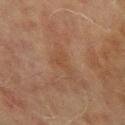Findings:
- image-analysis metrics · a within-lesion color-variation index near 0/10 and peripheral color asymmetry of about 0; a classifier nevus-likeness of about 0/100 and a detector confidence of about 100 out of 100 that the crop contains a lesion
- imaging modality · 15 mm crop, total-body photography
- tile lighting · cross-polarized
- lesion diameter · about 3 mm
- anatomic site · the left upper arm
- subject · male, roughly 70 years of age